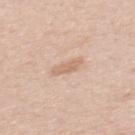Notes:
- biopsy status — total-body-photography surveillance lesion; no biopsy
- location — the mid back
- image — ~15 mm tile from a whole-body skin photo
- subject — female, roughly 40 years of age
- lesion diameter — ≈3 mm